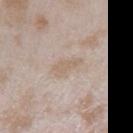illumination: white-light | diameter: ≈3.5 mm | location: the right forearm | acquisition: ~15 mm tile from a whole-body skin photo | patient: female, aged 23–27.